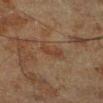Clinical impression: Imaged during a routine full-body skin examination; the lesion was not biopsied and no histopathology is available. Acquisition and patient details: The lesion is on the left lower leg. The patient is a male roughly 60 years of age. Automated tile analysis of the lesion measured a footprint of about 3.5 mm², an eccentricity of roughly 0.9, and two-axis asymmetry of about 0.4. The software also gave a lesion color around L≈30 a*≈17 b*≈23 in CIELAB and a normalized border contrast of about 5.5. It also reported a border-irregularity index near 4.5/10, internal color variation of about 0 on a 0–10 scale, and peripheral color asymmetry of about 0. A lesion tile, about 15 mm wide, cut from a 3D total-body photograph. Imaged with cross-polarized lighting. Measured at roughly 3 mm in maximum diameter.